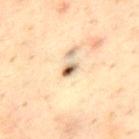biopsy status = total-body-photography surveillance lesion; no biopsy
diameter = about 2 mm
image source = 15 mm crop, total-body photography
patient = male, aged approximately 45
site = the upper back